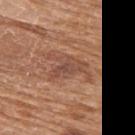Clinical impression: The lesion was photographed on a routine skin check and not biopsied; there is no pathology result. Clinical summary: A female subject, aged 68–72. Automated tile analysis of the lesion measured an average lesion color of about L≈49 a*≈22 b*≈29 (CIELAB), roughly 8 lightness units darker than nearby skin, and a normalized border contrast of about 6.5. And it measured a nevus-likeness score of about 10/100 and lesion-presence confidence of about 95/100. The lesion's longest dimension is about 5 mm. On the upper back. Imaged with white-light lighting. A roughly 15 mm field-of-view crop from a total-body skin photograph.